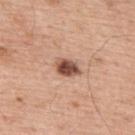Imaged during a routine full-body skin examination; the lesion was not biopsied and no histopathology is available.